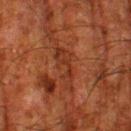Imaged during a routine full-body skin examination; the lesion was not biopsied and no histopathology is available.
A region of skin cropped from a whole-body photographic capture, roughly 15 mm wide.
The subject is a male aged around 80.
Longest diameter approximately 5 mm.
The total-body-photography lesion software estimated an area of roughly 6 mm², an eccentricity of roughly 0.95, and a shape-asymmetry score of about 0.55 (0 = symmetric). It also reported a lesion color around L≈27 a*≈23 b*≈28 in CIELAB and roughly 6 lightness units darker than nearby skin. The analysis additionally found lesion-presence confidence of about 70/100.
On the left thigh.